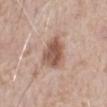notes=total-body-photography surveillance lesion; no biopsy
TBP lesion metrics=an eccentricity of roughly 0.7; a within-lesion color-variation index near 4/10
image source=15 mm crop, total-body photography
lighting=white-light illumination
diameter=about 4.5 mm
subject=male, in their 80s
location=the abdomen